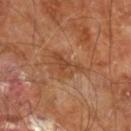biopsy_status: not biopsied; imaged during a skin examination
image:
  source: total-body photography crop
  field_of_view_mm: 15
lesion_size:
  long_diameter_mm_approx: 4.0
lighting: cross-polarized
site: right leg
patient:
  sex: male
  age_approx: 60